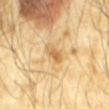biopsy_status: not biopsied; imaged during a skin examination
image:
  source: total-body photography crop
  field_of_view_mm: 15
site: mid back
automated_metrics:
  lesion_detection_confidence_0_100: 100
patient:
  sex: male
  age_approx: 65
lighting: cross-polarized
lesion_size:
  long_diameter_mm_approx: 3.0